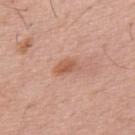Clinical impression: The lesion was tiled from a total-body skin photograph and was not biopsied. Clinical summary: The total-body-photography lesion software estimated a lesion color around L≈57 a*≈24 b*≈32 in CIELAB and a normalized border contrast of about 7. The analysis additionally found a nevus-likeness score of about 25/100 and a lesion-detection confidence of about 100/100. Imaged with white-light lighting. A male subject in their mid- to late 50s. This image is a 15 mm lesion crop taken from a total-body photograph. Measured at roughly 3 mm in maximum diameter.